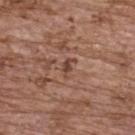Case summary:
• notes — imaged on a skin check; not biopsied
• automated metrics — border irregularity of about 4 on a 0–10 scale and radial color variation of about 0
• location — the upper back
• lesion size — ≈2.5 mm
• image source — ~15 mm crop, total-body skin-cancer survey
• illumination — white-light
• patient — female, aged 63 to 67